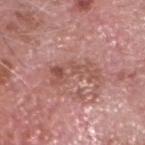The lesion was tiled from a total-body skin photograph and was not biopsied.
The lesion-visualizer software estimated a lesion color around L≈52 a*≈24 b*≈26 in CIELAB and roughly 8 lightness units darker than nearby skin. The software also gave an automated nevus-likeness rating near 0 out of 100.
The recorded lesion diameter is about 5.5 mm.
The lesion is on the head or neck.
A male patient aged 73 to 77.
A region of skin cropped from a whole-body photographic capture, roughly 15 mm wide.
This is a white-light tile.